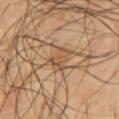biopsy_status: not biopsied; imaged during a skin examination
lesion_size:
  long_diameter_mm_approx: 3.0
site: chest
lighting: cross-polarized
automated_metrics:
  eccentricity: 0.7
  shape_asymmetry: 0.5
  cielab_L: 53
  cielab_a: 19
  cielab_b: 34
  vs_skin_contrast_norm: 6.0
  border_irregularity_0_10: 5.5
  color_variation_0_10: 4.0
  peripheral_color_asymmetry: 1.0
image:
  source: total-body photography crop
  field_of_view_mm: 15
patient:
  sex: male
  age_approx: 65